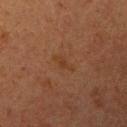follow-up = no biopsy performed (imaged during a skin exam); image source = ~15 mm tile from a whole-body skin photo; size = about 3 mm; lighting = cross-polarized; anatomic site = the left upper arm; patient = female, aged approximately 40.